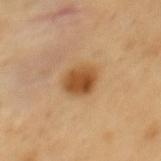biopsy status: imaged on a skin check; not biopsied | illumination: cross-polarized | acquisition: total-body-photography crop, ~15 mm field of view | image-analysis metrics: an average lesion color of about L≈51 a*≈21 b*≈39 (CIELAB), a lesion–skin lightness drop of about 13, and a normalized border contrast of about 9.5; a border-irregularity rating of about 1/10, a color-variation rating of about 6/10, and a peripheral color-asymmetry measure near 2; a detector confidence of about 100 out of 100 that the crop contains a lesion | location: the mid back | lesion diameter: ~3.5 mm (longest diameter) | patient: male, about 50 years old.